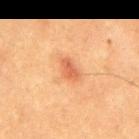Clinical impression:
This lesion was catalogued during total-body skin photography and was not selected for biopsy.
Acquisition and patient details:
A 15 mm close-up tile from a total-body photography series done for melanoma screening. The patient is a male aged around 50. On the back.